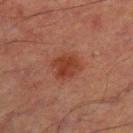Assessment: Imaged during a routine full-body skin examination; the lesion was not biopsied and no histopathology is available. Acquisition and patient details: A 15 mm close-up tile from a total-body photography series done for melanoma screening. The subject is a male aged 63–67. The lesion is located on the left thigh.